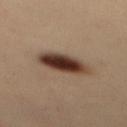Q: Was a biopsy performed?
A: total-body-photography surveillance lesion; no biopsy
Q: Who is the patient?
A: female, about 35 years old
Q: Lesion location?
A: the mid back
Q: What is the imaging modality?
A: ~15 mm crop, total-body skin-cancer survey